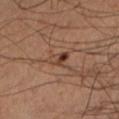Clinical impression: Imaged during a routine full-body skin examination; the lesion was not biopsied and no histopathology is available. Image and clinical context: A male patient, about 50 years old. The lesion is located on the left lower leg. A 15 mm crop from a total-body photograph taken for skin-cancer surveillance.